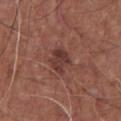  biopsy_status: not biopsied; imaged during a skin examination
  site: chest
  patient:
    sex: male
    age_approx: 65
  lighting: white-light
  lesion_size:
    long_diameter_mm_approx: 3.5
  image:
    source: total-body photography crop
    field_of_view_mm: 15